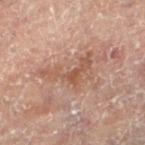No biopsy was performed on this lesion — it was imaged during a full skin examination and was not determined to be concerning.
A female patient aged 78–82.
A close-up tile cropped from a whole-body skin photograph, about 15 mm across.
The tile uses cross-polarized illumination.
From the right leg.
Approximately 6 mm at its widest.